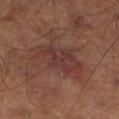Q: Was this lesion biopsied?
A: catalogued during a skin exam; not biopsied
Q: Lesion size?
A: ~6 mm (longest diameter)
Q: What is the anatomic site?
A: the right lower leg
Q: What kind of image is this?
A: 15 mm crop, total-body photography
Q: Patient demographics?
A: male, in their mid-60s
Q: Illumination type?
A: cross-polarized
Q: Automated lesion metrics?
A: an area of roughly 17 mm² and an eccentricity of roughly 0.8; a mean CIELAB color near L≈35 a*≈21 b*≈21, roughly 6 lightness units darker than nearby skin, and a lesion-to-skin contrast of about 6.5 (normalized; higher = more distinct); a border-irregularity rating of about 4/10, internal color variation of about 4.5 on a 0–10 scale, and a peripheral color-asymmetry measure near 1.5; a nevus-likeness score of about 30/100 and a lesion-detection confidence of about 100/100